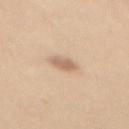Impression: Captured during whole-body skin photography for melanoma surveillance; the lesion was not biopsied. Context: A female patient approximately 30 years of age. The lesion is on the mid back. Cropped from a total-body skin-imaging series; the visible field is about 15 mm. Imaged with white-light lighting. Automated tile analysis of the lesion measured roughly 11 lightness units darker than nearby skin and a normalized lesion–skin contrast near 7. And it measured a border-irregularity index near 2.5/10 and internal color variation of about 3 on a 0–10 scale.